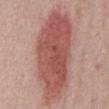Imaged with white-light lighting.
A region of skin cropped from a whole-body photographic capture, roughly 15 mm wide.
A male subject aged approximately 60.
From the chest.
Measured at roughly 12 mm in maximum diameter.